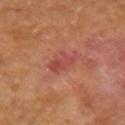Image and clinical context:
A female patient in their 60s. From the right upper arm. A 15 mm crop from a total-body photograph taken for skin-cancer surveillance. The lesion's longest dimension is about 3 mm. The tile uses cross-polarized illumination.